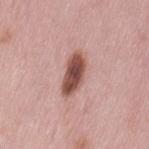Captured during whole-body skin photography for melanoma surveillance; the lesion was not biopsied. Imaged with white-light lighting. Measured at roughly 5 mm in maximum diameter. A female subject aged around 50. The lesion is located on the left thigh. A region of skin cropped from a whole-body photographic capture, roughly 15 mm wide. Automated image analysis of the tile measured an area of roughly 9 mm². The software also gave a lesion color around L≈51 a*≈23 b*≈25 in CIELAB, a lesion–skin lightness drop of about 17, and a normalized border contrast of about 11.5. The software also gave border irregularity of about 2.5 on a 0–10 scale, a color-variation rating of about 5/10, and peripheral color asymmetry of about 1.5. It also reported a classifier nevus-likeness of about 95/100 and lesion-presence confidence of about 100/100.